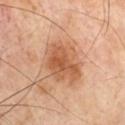Impression: Imaged during a routine full-body skin examination; the lesion was not biopsied and no histopathology is available. Acquisition and patient details: The lesion is on the chest. Automated image analysis of the tile measured a footprint of about 19 mm². And it measured a mean CIELAB color near L≈56 a*≈23 b*≈35 and a lesion–skin lightness drop of about 11. It also reported a nevus-likeness score of about 80/100 and a lesion-detection confidence of about 100/100. A male subject, about 70 years old. A lesion tile, about 15 mm wide, cut from a 3D total-body photograph. The lesion's longest dimension is about 5.5 mm.